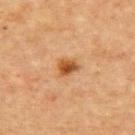Captured during whole-body skin photography for melanoma surveillance; the lesion was not biopsied.
A female patient, roughly 70 years of age.
A 15 mm close-up extracted from a 3D total-body photography capture.
The lesion is on the back.